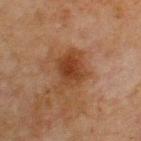Q: Is there a histopathology result?
A: catalogued during a skin exam; not biopsied